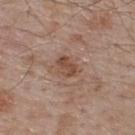| field | value |
|---|---|
| follow-up | total-body-photography surveillance lesion; no biopsy |
| subject | male, about 55 years old |
| automated metrics | an area of roughly 5 mm² and a symmetry-axis asymmetry near 0.25; a lesion color around L≈48 a*≈19 b*≈28 in CIELAB and a lesion-to-skin contrast of about 7 (normalized; higher = more distinct); a nevus-likeness score of about 20/100 and a detector confidence of about 100 out of 100 that the crop contains a lesion |
| imaging modality | ~15 mm tile from a whole-body skin photo |
| anatomic site | the upper back |
| illumination | white-light |